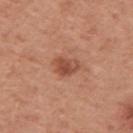Cropped from a whole-body photographic skin survey; the tile spans about 15 mm.
The subject is a male aged approximately 50.
The lesion is located on the upper back.